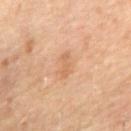Background:
Imaged with cross-polarized lighting. The lesion is on the abdomen. About 3 mm across. The subject is a male aged 68–72. A 15 mm close-up tile from a total-body photography series done for melanoma screening.